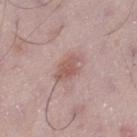This lesion was catalogued during total-body skin photography and was not selected for biopsy. This is a white-light tile. Approximately 3.5 mm at its widest. The subject is a male about 55 years old. A region of skin cropped from a whole-body photographic capture, roughly 15 mm wide. Located on the left thigh.